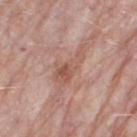Clinical impression: The lesion was photographed on a routine skin check and not biopsied; there is no pathology result. Background: Cropped from a total-body skin-imaging series; the visible field is about 15 mm. Longest diameter approximately 5.5 mm. Located on the right thigh. A female patient, aged approximately 70. Imaged with white-light lighting.